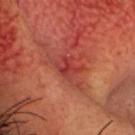Captured during whole-body skin photography for melanoma surveillance; the lesion was not biopsied. Cropped from a whole-body photographic skin survey; the tile spans about 15 mm. A male patient aged 48–52. From the head or neck. The tile uses cross-polarized illumination. Approximately 3 mm at its widest.